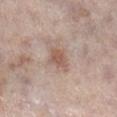Clinical impression: Recorded during total-body skin imaging; not selected for excision or biopsy. Context: Captured under white-light illumination. Cropped from a total-body skin-imaging series; the visible field is about 15 mm. Located on the right lower leg. Automated image analysis of the tile measured an area of roughly 4.5 mm² and a symmetry-axis asymmetry near 0.35. The analysis additionally found about 9 CIELAB-L* units darker than the surrounding skin and a normalized border contrast of about 7. And it measured a nevus-likeness score of about 65/100 and a lesion-detection confidence of about 100/100. Approximately 2.5 mm at its widest. A male patient aged 58 to 62.